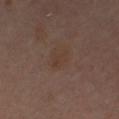Q: Was a biopsy performed?
A: imaged on a skin check; not biopsied
Q: How large is the lesion?
A: ~3 mm (longest diameter)
Q: What are the patient's age and sex?
A: female, in their 40s
Q: What kind of image is this?
A: ~15 mm crop, total-body skin-cancer survey
Q: What is the anatomic site?
A: the left leg